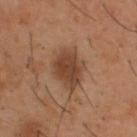Q: Automated lesion metrics?
A: an average lesion color of about L≈41 a*≈20 b*≈29 (CIELAB), a lesion–skin lightness drop of about 10, and a normalized border contrast of about 8.5; a border-irregularity index near 2.5/10, a color-variation rating of about 3/10, and radial color variation of about 1
Q: What are the patient's age and sex?
A: male, aged 38 to 42
Q: How was this image acquired?
A: 15 mm crop, total-body photography
Q: Lesion location?
A: the upper back
Q: How was the tile lit?
A: cross-polarized illumination
Q: What is the lesion's diameter?
A: about 4.5 mm